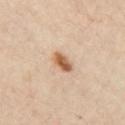{"biopsy_status": "not biopsied; imaged during a skin examination", "patient": {"age_approx": 55}, "lighting": "cross-polarized", "image": {"source": "total-body photography crop", "field_of_view_mm": 15}, "site": "chest", "automated_metrics": {"eccentricity": 0.8, "shape_asymmetry": 0.2, "cielab_L": 58, "cielab_a": 20, "cielab_b": 35, "vs_skin_contrast_norm": 10.0, "border_irregularity_0_10": 2.0, "color_variation_0_10": 4.0, "peripheral_color_asymmetry": 1.5}, "lesion_size": {"long_diameter_mm_approx": 3.0}}